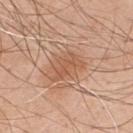Impression:
Part of a total-body skin-imaging series; this lesion was reviewed on a skin check and was not flagged for biopsy.
Clinical summary:
Measured at roughly 4 mm in maximum diameter. A 15 mm crop from a total-body photograph taken for skin-cancer surveillance. From the front of the torso. The tile uses white-light illumination. Automated image analysis of the tile measured a lesion area of about 6.5 mm², an outline eccentricity of about 0.8 (0 = round, 1 = elongated), and a symmetry-axis asymmetry near 0.4. The analysis additionally found a lesion color around L≈56 a*≈22 b*≈33 in CIELAB, a lesion–skin lightness drop of about 8, and a normalized border contrast of about 6. A male subject aged 48 to 52.